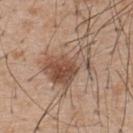Imaged during a routine full-body skin examination; the lesion was not biopsied and no histopathology is available. The tile uses white-light illumination. The lesion is located on the back. The patient is a male aged 53–57. Cropped from a total-body skin-imaging series; the visible field is about 15 mm.